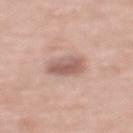Notes:
* biopsy status — catalogued during a skin exam; not biopsied
* patient — female, approximately 70 years of age
* body site — the back
* acquisition — ~15 mm crop, total-body skin-cancer survey